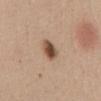The lesion was photographed on a routine skin check and not biopsied; there is no pathology result. Automated image analysis of the tile measured an area of roughly 5 mm² and two-axis asymmetry of about 0.15. The analysis additionally found a nevus-likeness score of about 100/100. Located on the abdomen. A female subject aged 48 to 52. A 15 mm crop from a total-body photograph taken for skin-cancer surveillance.